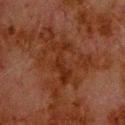| key | value |
|---|---|
| location | the upper back |
| imaging modality | ~15 mm tile from a whole-body skin photo |
| patient | male, aged approximately 80 |
| illumination | cross-polarized illumination |
| size | about 5.5 mm |
| automated metrics | a footprint of about 8 mm², an eccentricity of roughly 0.95, and a symmetry-axis asymmetry near 0.55; an average lesion color of about L≈23 a*≈21 b*≈26 (CIELAB) and a normalized border contrast of about 7; an automated nevus-likeness rating near 0 out of 100 and a detector confidence of about 95 out of 100 that the crop contains a lesion |